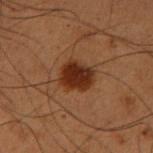Clinical impression: This lesion was catalogued during total-body skin photography and was not selected for biopsy. Image and clinical context: The total-body-photography lesion software estimated a normalized lesion–skin contrast near 12. A male patient in their mid-50s. Approximately 4 mm at its widest. From the right upper arm. A close-up tile cropped from a whole-body skin photograph, about 15 mm across. Imaged with cross-polarized lighting.